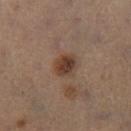{"automated_metrics": {"cielab_L": 38, "cielab_a": 17, "cielab_b": 25, "vs_skin_darker_L": 11.0, "vs_skin_contrast_norm": 9.5, "nevus_likeness_0_100": 90, "lesion_detection_confidence_0_100": 100}, "lighting": "cross-polarized", "image": {"source": "total-body photography crop", "field_of_view_mm": 15}, "site": "leg", "patient": {"sex": "male", "age_approx": 50}}